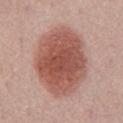Captured during whole-body skin photography for melanoma surveillance; the lesion was not biopsied.
On the front of the torso.
A male subject approximately 75 years of age.
The tile uses white-light illumination.
A roughly 15 mm field-of-view crop from a total-body skin photograph.